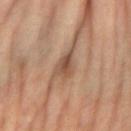Assessment: Imaged during a routine full-body skin examination; the lesion was not biopsied and no histopathology is available. Acquisition and patient details: Cropped from a whole-body photographic skin survey; the tile spans about 15 mm. The patient is a female about 65 years old. On the left forearm.